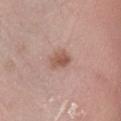Captured during whole-body skin photography for melanoma surveillance; the lesion was not biopsied. Located on the right lower leg. The lesion-visualizer software estimated an area of roughly 5 mm², an outline eccentricity of about 0.65 (0 = round, 1 = elongated), and a symmetry-axis asymmetry near 0.25. The software also gave a classifier nevus-likeness of about 70/100 and a lesion-detection confidence of about 100/100. A male subject, about 60 years old. Cropped from a total-body skin-imaging series; the visible field is about 15 mm. Measured at roughly 3 mm in maximum diameter. Captured under white-light illumination.